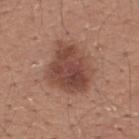Captured during whole-body skin photography for melanoma surveillance; the lesion was not biopsied.
The lesion is on the upper back.
The subject is a male aged 18 to 22.
This is a white-light tile.
Automated image analysis of the tile measured a footprint of about 23 mm², a shape eccentricity near 0.55, and two-axis asymmetry of about 0.25. And it measured about 11 CIELAB-L* units darker than the surrounding skin and a normalized lesion–skin contrast near 8.5. The software also gave a border-irregularity index near 2.5/10, a color-variation rating of about 4.5/10, and radial color variation of about 1.5. The software also gave a nevus-likeness score of about 70/100 and a lesion-detection confidence of about 100/100.
The recorded lesion diameter is about 6.5 mm.
This image is a 15 mm lesion crop taken from a total-body photograph.